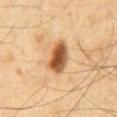| field | value |
|---|---|
| biopsy status | imaged on a skin check; not biopsied |
| automated lesion analysis | a lesion color around L≈44 a*≈19 b*≈33 in CIELAB and a normalized lesion–skin contrast near 12; a nevus-likeness score of about 100/100 and a detector confidence of about 100 out of 100 that the crop contains a lesion |
| location | the abdomen |
| lesion size | about 4 mm |
| illumination | cross-polarized illumination |
| image | ~15 mm crop, total-body skin-cancer survey |
| patient | male, in their mid-60s |